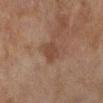Assessment:
The lesion was tiled from a total-body skin photograph and was not biopsied.
Context:
A female subject aged 58–62. A 15 mm close-up extracted from a 3D total-body photography capture. From the left lower leg. The total-body-photography lesion software estimated a footprint of about 5 mm², a shape eccentricity near 0.5, and two-axis asymmetry of about 0.15. Imaged with cross-polarized lighting.